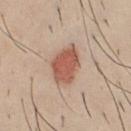* notes · imaged on a skin check; not biopsied